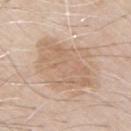The lesion was photographed on a routine skin check and not biopsied; there is no pathology result.
This is a white-light tile.
The patient is a male aged around 70.
The lesion is located on the left upper arm.
A close-up tile cropped from a whole-body skin photograph, about 15 mm across.